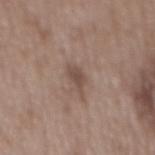Imaged during a routine full-body skin examination; the lesion was not biopsied and no histopathology is available. A male subject, in their 50s. A 15 mm close-up extracted from a 3D total-body photography capture. The lesion is on the mid back. Approximately 3.5 mm at its widest. The tile uses white-light illumination.